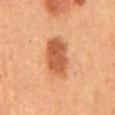Impression:
Recorded during total-body skin imaging; not selected for excision or biopsy.
Image and clinical context:
Located on the back. A lesion tile, about 15 mm wide, cut from a 3D total-body photograph. The total-body-photography lesion software estimated a lesion-to-skin contrast of about 8.5 (normalized; higher = more distinct). And it measured a border-irregularity rating of about 2.5/10 and internal color variation of about 3.5 on a 0–10 scale. The lesion's longest dimension is about 6 mm. The patient is female. Captured under cross-polarized illumination.